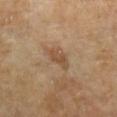Notes:
– anatomic site — the arm
– image-analysis metrics — a lesion-detection confidence of about 100/100
– image — 15 mm crop, total-body photography
– lesion size — about 3 mm
– subject — female, aged 63 to 67
– tile lighting — cross-polarized